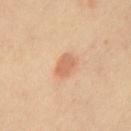* biopsy status: catalogued during a skin exam; not biopsied
* lesion diameter: ~2.5 mm (longest diameter)
* image: total-body-photography crop, ~15 mm field of view
* site: the chest
* TBP lesion metrics: a mean CIELAB color near L≈65 a*≈24 b*≈36, a lesion–skin lightness drop of about 10, and a lesion-to-skin contrast of about 6.5 (normalized; higher = more distinct); border irregularity of about 1.5 on a 0–10 scale, a within-lesion color-variation index near 1.5/10, and radial color variation of about 0.5
* lighting: cross-polarized